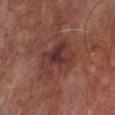Recorded during total-body skin imaging; not selected for excision or biopsy. Longest diameter approximately 5 mm. A 15 mm close-up extracted from a 3D total-body photography capture. A male subject, in their mid- to late 60s. On the front of the torso. The tile uses white-light illumination.